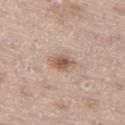Located on the left thigh. About 3 mm across. Cropped from a total-body skin-imaging series; the visible field is about 15 mm. A male subject aged approximately 65.